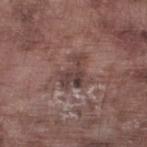<lesion>
  <biopsy_status>not biopsied; imaged during a skin examination</biopsy_status>
  <image>
    <source>total-body photography crop</source>
    <field_of_view_mm>15</field_of_view_mm>
  </image>
  <site>left lower leg</site>
  <lighting>white-light</lighting>
  <patient>
    <sex>male</sex>
    <age_approx>75</age_approx>
  </patient>
  <automated_metrics>
    <area_mm2_approx>8.5</area_mm2_approx>
    <eccentricity>0.65</eccentricity>
    <shape_asymmetry>0.55</shape_asymmetry>
    <vs_skin_darker_L>9.0</vs_skin_darker_L>
  </automated_metrics>
  <lesion_size>
    <long_diameter_mm_approx>4.0</long_diameter_mm_approx>
  </lesion_size>
</lesion>